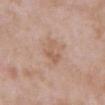Imaged during a routine full-body skin examination; the lesion was not biopsied and no histopathology is available. The total-body-photography lesion software estimated a shape eccentricity near 0.8 and a symmetry-axis asymmetry near 0.45. The software also gave border irregularity of about 5 on a 0–10 scale, a within-lesion color-variation index near 1/10, and peripheral color asymmetry of about 0.5. The software also gave a detector confidence of about 100 out of 100 that the crop contains a lesion. The lesion is located on the abdomen. A region of skin cropped from a whole-body photographic capture, roughly 15 mm wide. The lesion's longest dimension is about 3.5 mm. A male subject aged 53 to 57. Imaged with white-light lighting.